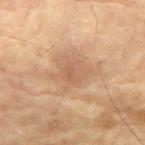Part of a total-body skin-imaging series; this lesion was reviewed on a skin check and was not flagged for biopsy. An algorithmic analysis of the crop reported a footprint of about 15 mm², an outline eccentricity of about 0.6 (0 = round, 1 = elongated), and a symmetry-axis asymmetry near 0.25. The software also gave about 7 CIELAB-L* units darker than the surrounding skin and a normalized border contrast of about 5. Imaged with cross-polarized lighting. Cropped from a total-body skin-imaging series; the visible field is about 15 mm. The lesion is on the right upper arm. Longest diameter approximately 5.5 mm. A male subject aged 83 or over.